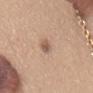No biopsy was performed on this lesion — it was imaged during a full skin examination and was not determined to be concerning.
Located on the chest.
A female patient, aged 38–42.
A 15 mm crop from a total-body photograph taken for skin-cancer surveillance.